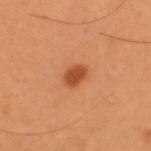The lesion was tiled from a total-body skin photograph and was not biopsied.
From the back.
A male subject, in their mid- to late 50s.
This image is a 15 mm lesion crop taken from a total-body photograph.
Approximately 2.5 mm at its widest.